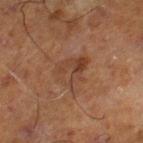<tbp_lesion>
  <biopsy_status>not biopsied; imaged during a skin examination</biopsy_status>
  <lighting>cross-polarized</lighting>
  <patient>
    <sex>male</sex>
    <age_approx>70</age_approx>
  </patient>
  <site>right lower leg</site>
  <lesion_size>
    <long_diameter_mm_approx>4.5</long_diameter_mm_approx>
  </lesion_size>
  <image>
    <source>total-body photography crop</source>
    <field_of_view_mm>15</field_of_view_mm>
  </image>
</tbp_lesion>